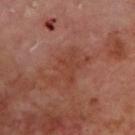Assessment: This lesion was catalogued during total-body skin photography and was not selected for biopsy. Clinical summary: From the upper back. A male subject, approximately 70 years of age. Approximately 5.5 mm at its widest. The lesion-visualizer software estimated an area of roughly 12 mm² and a symmetry-axis asymmetry near 0.55. It also reported an automated nevus-likeness rating near 0 out of 100 and a lesion-detection confidence of about 100/100. Captured under cross-polarized illumination. A lesion tile, about 15 mm wide, cut from a 3D total-body photograph.